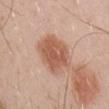workup: total-body-photography surveillance lesion; no biopsy | automated metrics: a lesion area of about 16 mm², an outline eccentricity of about 0.7 (0 = round, 1 = elongated), and two-axis asymmetry of about 0.2; an average lesion color of about L≈57 a*≈22 b*≈31 (CIELAB), about 12 CIELAB-L* units darker than the surrounding skin, and a lesion-to-skin contrast of about 8.5 (normalized; higher = more distinct); a border-irregularity rating of about 2.5/10, a within-lesion color-variation index near 3/10, and a peripheral color-asymmetry measure near 1; an automated nevus-likeness rating near 80 out of 100 | subject: male, aged approximately 30 | location: the right upper arm | illumination: white-light illumination | size: about 5.5 mm | image source: ~15 mm tile from a whole-body skin photo.